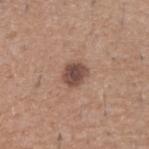Q: Was this lesion biopsied?
A: total-body-photography surveillance lesion; no biopsy
Q: How was the tile lit?
A: white-light
Q: Patient demographics?
A: male, about 50 years old
Q: Lesion size?
A: ≈2.5 mm
Q: Automated lesion metrics?
A: a lesion area of about 5.5 mm², an outline eccentricity of about 0.5 (0 = round, 1 = elongated), and a symmetry-axis asymmetry near 0.15; a lesion color around L≈47 a*≈19 b*≈24 in CIELAB, a lesion–skin lightness drop of about 14, and a lesion-to-skin contrast of about 10 (normalized; higher = more distinct); a border-irregularity rating of about 1.5/10, internal color variation of about 3 on a 0–10 scale, and peripheral color asymmetry of about 1; a nevus-likeness score of about 30/100 and a lesion-detection confidence of about 100/100
Q: What is the imaging modality?
A: 15 mm crop, total-body photography
Q: What is the anatomic site?
A: the right upper arm